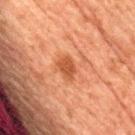follow-up — imaged on a skin check; not biopsied
TBP lesion metrics — a footprint of about 4.5 mm², an eccentricity of roughly 0.7, and a shape-asymmetry score of about 0.25 (0 = symmetric); an average lesion color of about L≈42 a*≈25 b*≈33 (CIELAB) and roughly 9 lightness units darker than nearby skin; border irregularity of about 2.5 on a 0–10 scale, internal color variation of about 1.5 on a 0–10 scale, and peripheral color asymmetry of about 0.5
imaging modality — ~15 mm tile from a whole-body skin photo
patient — male, aged around 80
location — the lower back
lighting — cross-polarized
size — ≈3 mm